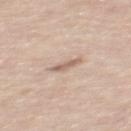Captured during whole-body skin photography for melanoma surveillance; the lesion was not biopsied.
A 15 mm crop from a total-body photograph taken for skin-cancer surveillance.
The lesion is located on the upper back.
The patient is a male about 55 years old.